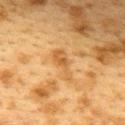Part of a total-body skin-imaging series; this lesion was reviewed on a skin check and was not flagged for biopsy. A 15 mm crop from a total-body photograph taken for skin-cancer surveillance. Longest diameter approximately 4.5 mm. The tile uses cross-polarized illumination. The subject is a female aged around 40. Located on the upper back.